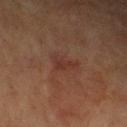Q: Is there a histopathology result?
A: imaged on a skin check; not biopsied
Q: How was the tile lit?
A: cross-polarized
Q: Who is the patient?
A: female, aged around 80
Q: What is the imaging modality?
A: ~15 mm tile from a whole-body skin photo
Q: What did automated image analysis measure?
A: a border-irregularity rating of about 5.5/10, internal color variation of about 2 on a 0–10 scale, and peripheral color asymmetry of about 0.5; a classifier nevus-likeness of about 0/100
Q: Where on the body is the lesion?
A: the left upper arm